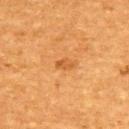Context: The subject is a male aged 58–62. The tile uses cross-polarized illumination. A roughly 15 mm field-of-view crop from a total-body skin photograph. The lesion is located on the upper back. The lesion's longest dimension is about 2 mm.